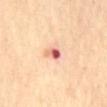follow-up — imaged on a skin check; not biopsied
lesion size — about 2.5 mm
subject — female, aged around 65
imaging modality — 15 mm crop, total-body photography
location — the mid back